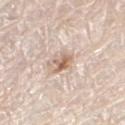Q: Was a biopsy performed?
A: catalogued during a skin exam; not biopsied
Q: What are the patient's age and sex?
A: male, in their 80s
Q: How was this image acquired?
A: ~15 mm crop, total-body skin-cancer survey
Q: Where on the body is the lesion?
A: the right thigh
Q: Illumination type?
A: white-light
Q: Lesion size?
A: ~3 mm (longest diameter)